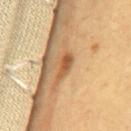Imaged during a routine full-body skin examination; the lesion was not biopsied and no histopathology is available.
The patient is a female approximately 40 years of age.
Cropped from a whole-body photographic skin survey; the tile spans about 15 mm.
Approximately 3 mm at its widest.
The lesion is located on the mid back.
Automated tile analysis of the lesion measured a footprint of about 4 mm² and an eccentricity of roughly 0.85. The software also gave a mean CIELAB color near L≈55 a*≈22 b*≈39, roughly 10 lightness units darker than nearby skin, and a normalized lesion–skin contrast near 7.5. It also reported a classifier nevus-likeness of about 95/100 and a detector confidence of about 100 out of 100 that the crop contains a lesion.